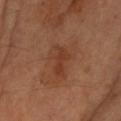biopsy status: total-body-photography surveillance lesion; no biopsy | subject: female, aged 58–62 | acquisition: 15 mm crop, total-body photography | site: the left forearm.